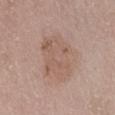{
  "biopsy_status": "not biopsied; imaged during a skin examination",
  "lighting": "white-light",
  "image": {
    "source": "total-body photography crop",
    "field_of_view_mm": 15
  },
  "site": "front of the torso",
  "patient": {
    "sex": "female",
    "age_approx": "85+"
  },
  "lesion_size": {
    "long_diameter_mm_approx": 6.0
  }
}